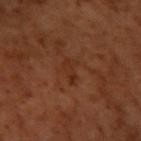* workup — catalogued during a skin exam; not biopsied
* subject — male, aged 58–62
* size — ≈3 mm
* site — the arm
* image — ~15 mm tile from a whole-body skin photo